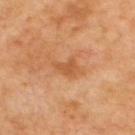Captured during whole-body skin photography for melanoma surveillance; the lesion was not biopsied. Captured under cross-polarized illumination. A roughly 15 mm field-of-view crop from a total-body skin photograph. A patient roughly 65 years of age. From the upper back.